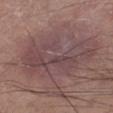workup: catalogued during a skin exam; not biopsied
patient: male, aged 43–47
anatomic site: the right lower leg
imaging modality: 15 mm crop, total-body photography